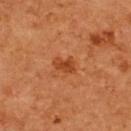Q: Was a biopsy performed?
A: total-body-photography surveillance lesion; no biopsy
Q: What did automated image analysis measure?
A: an area of roughly 4 mm², an outline eccentricity of about 0.75 (0 = round, 1 = elongated), and a shape-asymmetry score of about 0.25 (0 = symmetric); an average lesion color of about L≈45 a*≈30 b*≈41 (CIELAB), roughly 9 lightness units darker than nearby skin, and a normalized lesion–skin contrast near 7.5; a nevus-likeness score of about 80/100 and a lesion-detection confidence of about 100/100
Q: Lesion location?
A: the upper back
Q: Who is the patient?
A: female, in their mid- to late 60s
Q: How was this image acquired?
A: total-body-photography crop, ~15 mm field of view
Q: How was the tile lit?
A: cross-polarized illumination
Q: Lesion size?
A: ~3 mm (longest diameter)